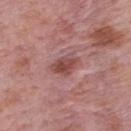notes: catalogued during a skin exam; not biopsied
anatomic site: the mid back
TBP lesion metrics: a mean CIELAB color near L≈48 a*≈25 b*≈23, roughly 10 lightness units darker than nearby skin, and a lesion-to-skin contrast of about 7.5 (normalized; higher = more distinct); border irregularity of about 2.5 on a 0–10 scale and a within-lesion color-variation index near 4/10; a lesion-detection confidence of about 100/100
tile lighting: white-light
size: ~3.5 mm (longest diameter)
acquisition: total-body-photography crop, ~15 mm field of view
subject: male, aged 73 to 77